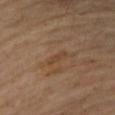biopsy status: catalogued during a skin exam; not biopsied | patient: female, approximately 45 years of age | body site: the left forearm | image source: total-body-photography crop, ~15 mm field of view.